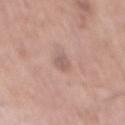No biopsy was performed on this lesion — it was imaged during a full skin examination and was not determined to be concerning.
The patient is a male approximately 60 years of age.
The lesion is on the mid back.
The lesion's longest dimension is about 2.5 mm.
A 15 mm close-up tile from a total-body photography series done for melanoma screening.